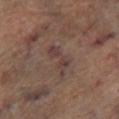The lesion was photographed on a routine skin check and not biopsied; there is no pathology result.
On the left lower leg.
A male subject aged 63 to 67.
A region of skin cropped from a whole-body photographic capture, roughly 15 mm wide.